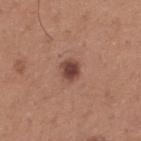Recorded during total-body skin imaging; not selected for excision or biopsy. Located on the upper back. A male patient aged 63–67. A lesion tile, about 15 mm wide, cut from a 3D total-body photograph. The tile uses white-light illumination. Automated tile analysis of the lesion measured an area of roughly 5 mm², a shape eccentricity near 0.5, and a symmetry-axis asymmetry near 0.2. The software also gave a classifier nevus-likeness of about 95/100 and a lesion-detection confidence of about 100/100.